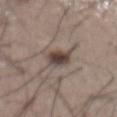No biopsy was performed on this lesion — it was imaged during a full skin examination and was not determined to be concerning. The lesion is located on the abdomen. Cropped from a whole-body photographic skin survey; the tile spans about 15 mm. The patient is a male aged 53–57. This is a white-light tile. The lesion-visualizer software estimated a lesion area of about 7 mm² and a shape eccentricity near 0.8. And it measured a lesion color around L≈43 a*≈13 b*≈19 in CIELAB and about 14 CIELAB-L* units darker than the surrounding skin. It also reported internal color variation of about 6.5 on a 0–10 scale and peripheral color asymmetry of about 2. The analysis additionally found a classifier nevus-likeness of about 100/100 and a detector confidence of about 100 out of 100 that the crop contains a lesion. Approximately 4 mm at its widest.